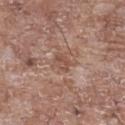Recorded during total-body skin imaging; not selected for excision or biopsy. The lesion-visualizer software estimated an area of roughly 3.5 mm² and a symmetry-axis asymmetry near 0.25. The software also gave a lesion color around L≈50 a*≈20 b*≈27 in CIELAB and a normalized lesion–skin contrast near 5.5. It also reported border irregularity of about 2.5 on a 0–10 scale, a within-lesion color-variation index near 2.5/10, and peripheral color asymmetry of about 1. The analysis additionally found a nevus-likeness score of about 0/100 and a lesion-detection confidence of about 95/100. A male subject, aged approximately 70. The lesion is located on the chest. Longest diameter approximately 2.5 mm. The tile uses white-light illumination. Cropped from a total-body skin-imaging series; the visible field is about 15 mm.